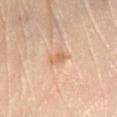Acquisition and patient details:
Measured at roughly 2 mm in maximum diameter. Cropped from a total-body skin-imaging series; the visible field is about 15 mm. On the left lower leg. The subject is a female aged around 65. Imaged with cross-polarized lighting. The total-body-photography lesion software estimated an area of roughly 2.5 mm², an eccentricity of roughly 0.8, and a symmetry-axis asymmetry near 0.3. The software also gave about 9 CIELAB-L* units darker than the surrounding skin and a normalized border contrast of about 6.5. It also reported a nevus-likeness score of about 15/100 and a detector confidence of about 100 out of 100 that the crop contains a lesion.